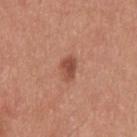Assessment: Captured during whole-body skin photography for melanoma surveillance; the lesion was not biopsied. Acquisition and patient details: Automated tile analysis of the lesion measured an automated nevus-likeness rating near 85 out of 100 and a lesion-detection confidence of about 100/100. Cropped from a total-body skin-imaging series; the visible field is about 15 mm. On the upper back. The lesion's longest dimension is about 3 mm. The patient is a male aged around 30. Imaged with white-light lighting.